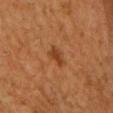- follow-up · no biopsy performed (imaged during a skin exam)
- size · ≈3 mm
- site · the head or neck
- illumination · cross-polarized
- patient · male, aged approximately 70
- image source · ~15 mm tile from a whole-body skin photo
- TBP lesion metrics · lesion-presence confidence of about 100/100